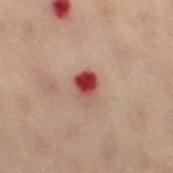body site: the left leg | patient: female, aged around 60 | acquisition: 15 mm crop, total-body photography | automated metrics: an area of roughly 5.5 mm², an outline eccentricity of about 0.7 (0 = round, 1 = elongated), and two-axis asymmetry of about 0.15; border irregularity of about 1.5 on a 0–10 scale, internal color variation of about 10 on a 0–10 scale, and peripheral color asymmetry of about 4.5 | lighting: cross-polarized | size: about 3.5 mm.